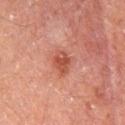biopsy status = total-body-photography surveillance lesion; no biopsy
acquisition = total-body-photography crop, ~15 mm field of view
body site = the mid back
patient = male, aged 58 to 62
lesion diameter = ≈3 mm
illumination = cross-polarized illumination